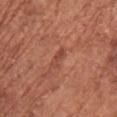Notes:
• biopsy status — catalogued during a skin exam; not biopsied
• image — ~15 mm crop, total-body skin-cancer survey
• body site — the upper back
• lesion size — ≈3 mm
• subject — female, aged around 75